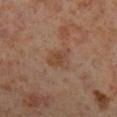<case>
<biopsy_status>not biopsied; imaged during a skin examination</biopsy_status>
<site>right lower leg</site>
<automated_metrics>
  <nevus_likeness_0_100>0</nevus_likeness_0_100>
  <lesion_detection_confidence_0_100>100</lesion_detection_confidence_0_100>
</automated_metrics>
<lighting>cross-polarized</lighting>
<patient>
  <sex>male</sex>
  <age_approx>60</age_approx>
</patient>
<image>
  <source>total-body photography crop</source>
  <field_of_view_mm>15</field_of_view_mm>
</image>
</case>